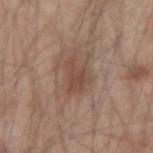Captured during whole-body skin photography for melanoma surveillance; the lesion was not biopsied.
The subject is a male aged around 45.
The tile uses white-light illumination.
Automated tile analysis of the lesion measured an outline eccentricity of about 0.8 (0 = round, 1 = elongated) and a shape-asymmetry score of about 0.45 (0 = symmetric). The analysis additionally found a color-variation rating of about 2/10 and a peripheral color-asymmetry measure near 0.5.
Cropped from a whole-body photographic skin survey; the tile spans about 15 mm.
The recorded lesion diameter is about 4 mm.
On the right forearm.